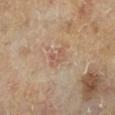Captured during whole-body skin photography for melanoma surveillance; the lesion was not biopsied. A male subject aged 63 to 67. The lesion is on the left lower leg. A region of skin cropped from a whole-body photographic capture, roughly 15 mm wide. The recorded lesion diameter is about 3 mm. Captured under cross-polarized illumination.